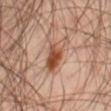Clinical impression: No biopsy was performed on this lesion — it was imaged during a full skin examination and was not determined to be concerning. Acquisition and patient details: Captured under cross-polarized illumination. The subject is a male about 45 years old. Approximately 4.5 mm at its widest. From the right thigh. The lesion-visualizer software estimated a lesion area of about 8.5 mm², a shape eccentricity near 0.85, and a shape-asymmetry score of about 0.3 (0 = symmetric). The software also gave a nevus-likeness score of about 95/100 and lesion-presence confidence of about 100/100. A 15 mm crop from a total-body photograph taken for skin-cancer surveillance.